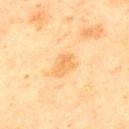Acquisition and patient details:
The subject is a male approximately 45 years of age. A lesion tile, about 15 mm wide, cut from a 3D total-body photograph. Automated image analysis of the tile measured a detector confidence of about 100 out of 100 that the crop contains a lesion. From the upper back.